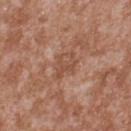This lesion was catalogued during total-body skin photography and was not selected for biopsy. A male subject approximately 45 years of age. This image is a 15 mm lesion crop taken from a total-body photograph. The lesion is on the back. The tile uses white-light illumination.